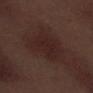workup: catalogued during a skin exam; not biopsied | anatomic site: the right lower leg | acquisition: total-body-photography crop, ~15 mm field of view | subject: male, aged approximately 70.